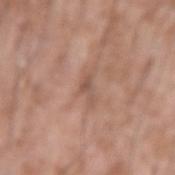– workup: no biopsy performed (imaged during a skin exam)
– subject: male, aged around 60
– illumination: white-light illumination
– lesion diameter: ~2.5 mm (longest diameter)
– imaging modality: 15 mm crop, total-body photography
– anatomic site: the left forearm
– automated metrics: a lesion area of about 2.5 mm², an outline eccentricity of about 0.85 (0 = round, 1 = elongated), and a shape-asymmetry score of about 0.65 (0 = symmetric); a lesion color around L≈53 a*≈20 b*≈26 in CIELAB, about 8 CIELAB-L* units darker than the surrounding skin, and a normalized border contrast of about 5.5; a border-irregularity rating of about 7/10 and peripheral color asymmetry of about 0; a nevus-likeness score of about 0/100 and a detector confidence of about 95 out of 100 that the crop contains a lesion